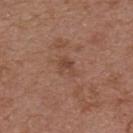Notes:
• site: the front of the torso
• patient: male, about 55 years old
• size: about 2.5 mm
• image-analysis metrics: an average lesion color of about L≈45 a*≈21 b*≈28 (CIELAB) and a lesion-to-skin contrast of about 5.5 (normalized; higher = more distinct); a classifier nevus-likeness of about 0/100 and a detector confidence of about 100 out of 100 that the crop contains a lesion
• image source: ~15 mm crop, total-body skin-cancer survey
• lighting: white-light illumination